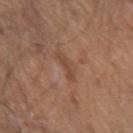Notes:
• biopsy status: catalogued during a skin exam; not biopsied
• image-analysis metrics: an area of roughly 3 mm², an outline eccentricity of about 0.9 (0 = round, 1 = elongated), and a shape-asymmetry score of about 0.35 (0 = symmetric); a mean CIELAB color near L≈46 a*≈20 b*≈28 and a normalized border contrast of about 5.5
• illumination: white-light
• image source: ~15 mm tile from a whole-body skin photo
• location: the left forearm
• lesion diameter: ~3 mm (longest diameter)
• subject: male, aged approximately 70